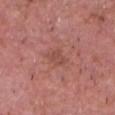The lesion was photographed on a routine skin check and not biopsied; there is no pathology result.
The lesion's longest dimension is about 2.5 mm.
The lesion is located on the head or neck.
Captured under white-light illumination.
A roughly 15 mm field-of-view crop from a total-body skin photograph.
A male subject, aged 58–62.